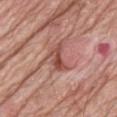This lesion was catalogued during total-body skin photography and was not selected for biopsy. The subject is a male roughly 80 years of age. The lesion's longest dimension is about 3.5 mm. The tile uses white-light illumination. From the back. A 15 mm crop from a total-body photograph taken for skin-cancer surveillance.